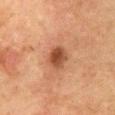Findings:
• automated lesion analysis: a lesion color around L≈40 a*≈21 b*≈29 in CIELAB, about 11 CIELAB-L* units darker than the surrounding skin, and a lesion-to-skin contrast of about 9 (normalized; higher = more distinct)
• lighting: cross-polarized
• patient: female, aged 58–62
• location: the chest
• image source: 15 mm crop, total-body photography
• lesion size: about 3 mm